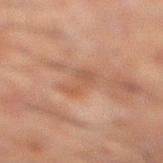A male patient aged around 60.
The lesion is on the left leg.
Cropped from a whole-body photographic skin survey; the tile spans about 15 mm.